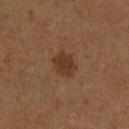Impression:
Captured during whole-body skin photography for melanoma surveillance; the lesion was not biopsied.
Image and clinical context:
From the left lower leg. Measured at roughly 3 mm in maximum diameter. The lesion-visualizer software estimated a footprint of about 6 mm² and an outline eccentricity of about 0.5 (0 = round, 1 = elongated). The analysis additionally found border irregularity of about 2 on a 0–10 scale and internal color variation of about 2 on a 0–10 scale. It also reported an automated nevus-likeness rating near 60 out of 100 and a detector confidence of about 100 out of 100 that the crop contains a lesion. The subject is a male aged 73–77. Captured under cross-polarized illumination. A roughly 15 mm field-of-view crop from a total-body skin photograph.